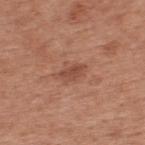The lesion was tiled from a total-body skin photograph and was not biopsied.
A male patient roughly 55 years of age.
Captured under white-light illumination.
An algorithmic analysis of the crop reported a mean CIELAB color near L≈48 a*≈24 b*≈29 and about 9 CIELAB-L* units darker than the surrounding skin.
A region of skin cropped from a whole-body photographic capture, roughly 15 mm wide.
The recorded lesion diameter is about 3 mm.
The lesion is on the upper back.